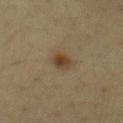biopsy status = imaged on a skin check; not biopsied
diameter = ~2.5 mm (longest diameter)
illumination = cross-polarized illumination
site = the abdomen
image-analysis metrics = an average lesion color of about L≈37 a*≈13 b*≈28 (CIELAB), roughly 9 lightness units darker than nearby skin, and a normalized lesion–skin contrast near 9; a border-irregularity index near 1.5/10
subject = male, roughly 35 years of age
image = ~15 mm crop, total-body skin-cancer survey